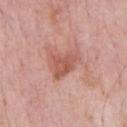Notes:
– follow-up — no biopsy performed (imaged during a skin exam)
– acquisition — 15 mm crop, total-body photography
– illumination — white-light illumination
– patient — male, approximately 60 years of age
– automated metrics — a lesion color around L≈56 a*≈26 b*≈28 in CIELAB, a lesion–skin lightness drop of about 10, and a lesion-to-skin contrast of about 7 (normalized; higher = more distinct); a border-irregularity rating of about 4/10, a within-lesion color-variation index near 2.5/10, and peripheral color asymmetry of about 1; a classifier nevus-likeness of about 5/100 and a lesion-detection confidence of about 100/100
– lesion size — ~4 mm (longest diameter)
– body site — the chest